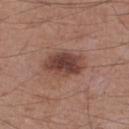Q: Was this lesion biopsied?
A: total-body-photography surveillance lesion; no biopsy
Q: How was this image acquired?
A: ~15 mm tile from a whole-body skin photo
Q: Where on the body is the lesion?
A: the leg
Q: Patient demographics?
A: male, in their mid-50s
Q: How large is the lesion?
A: ~4.5 mm (longest diameter)
Q: How was the tile lit?
A: white-light